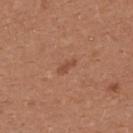| field | value |
|---|---|
| notes | no biopsy performed (imaged during a skin exam) |
| illumination | white-light illumination |
| subject | female, roughly 25 years of age |
| diameter | about 2.5 mm |
| image | ~15 mm tile from a whole-body skin photo |
| anatomic site | the upper back |